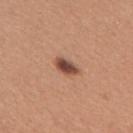Assessment: No biopsy was performed on this lesion — it was imaged during a full skin examination and was not determined to be concerning. Image and clinical context: About 3 mm across. A female patient, aged 28 to 32. The lesion is located on the upper back. Captured under white-light illumination. Automated tile analysis of the lesion measured a footprint of about 4 mm², an eccentricity of roughly 0.85, and a symmetry-axis asymmetry near 0.25. And it measured an average lesion color of about L≈47 a*≈22 b*≈28 (CIELAB), about 16 CIELAB-L* units darker than the surrounding skin, and a normalized border contrast of about 11. And it measured border irregularity of about 2.5 on a 0–10 scale, internal color variation of about 4.5 on a 0–10 scale, and radial color variation of about 1.5. It also reported a classifier nevus-likeness of about 100/100 and lesion-presence confidence of about 100/100. A lesion tile, about 15 mm wide, cut from a 3D total-body photograph.